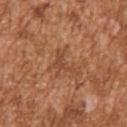Q: Is there a histopathology result?
A: no biopsy performed (imaged during a skin exam)
Q: Where on the body is the lesion?
A: the right upper arm
Q: What are the patient's age and sex?
A: male, aged 43 to 47
Q: How was this image acquired?
A: total-body-photography crop, ~15 mm field of view